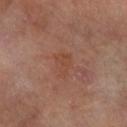| feature | finding |
|---|---|
| biopsy status | catalogued during a skin exam; not biopsied |
| patient | female, aged 63 to 67 |
| acquisition | ~15 mm tile from a whole-body skin photo |
| tile lighting | cross-polarized |
| body site | the left arm |
| diameter | ~3 mm (longest diameter) |